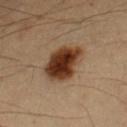Acquisition and patient details: A male subject approximately 55 years of age. Cropped from a whole-body photographic skin survey; the tile spans about 15 mm. Automated tile analysis of the lesion measured a lesion area of about 15 mm², an outline eccentricity of about 0.45 (0 = round, 1 = elongated), and a shape-asymmetry score of about 0.15 (0 = symmetric). It also reported an average lesion color of about L≈28 a*≈17 b*≈25 (CIELAB), roughly 15 lightness units darker than nearby skin, and a lesion-to-skin contrast of about 14 (normalized; higher = more distinct). On the left forearm. The lesion's longest dimension is about 4.5 mm.